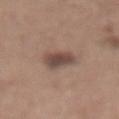<record>
  <biopsy_status>not biopsied; imaged during a skin examination</biopsy_status>
  <automated_metrics>
    <eccentricity>0.45</eccentricity>
    <cielab_L>47</cielab_L>
    <cielab_a>16</cielab_a>
    <cielab_b>22</cielab_b>
    <vs_skin_darker_L>11.0</vs_skin_darker_L>
    <border_irregularity_0_10>2.0</border_irregularity_0_10>
    <peripheral_color_asymmetry>1.5</peripheral_color_asymmetry>
  </automated_metrics>
  <lesion_size>
    <long_diameter_mm_approx>3.5</long_diameter_mm_approx>
  </lesion_size>
  <patient>
    <sex>female</sex>
    <age_approx>65</age_approx>
  </patient>
  <lighting>white-light</lighting>
  <image>
    <source>total-body photography crop</source>
    <field_of_view_mm>15</field_of_view_mm>
  </image>
  <site>abdomen</site>
</record>